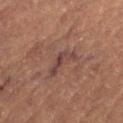{"biopsy_status": "not biopsied; imaged during a skin examination", "lighting": "cross-polarized", "lesion_size": {"long_diameter_mm_approx": 4.0}, "image": {"source": "total-body photography crop", "field_of_view_mm": 15}, "patient": {"sex": "female", "age_approx": 70}, "site": "left thigh"}